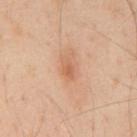<lesion>
  <biopsy_status>not biopsied; imaged during a skin examination</biopsy_status>
  <patient>
    <sex>male</sex>
    <age_approx>45</age_approx>
  </patient>
  <site>mid back</site>
  <image>
    <source>total-body photography crop</source>
    <field_of_view_mm>15</field_of_view_mm>
  </image>
</lesion>